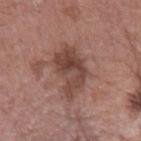Assessment:
Captured during whole-body skin photography for melanoma surveillance; the lesion was not biopsied.
Background:
A 15 mm close-up extracted from a 3D total-body photography capture. A male subject aged approximately 75. Located on the arm.